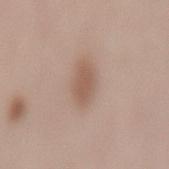{
  "biopsy_status": "not biopsied; imaged during a skin examination",
  "lighting": "white-light",
  "image": {
    "source": "total-body photography crop",
    "field_of_view_mm": 15
  },
  "site": "mid back",
  "lesion_size": {
    "long_diameter_mm_approx": 3.0
  },
  "patient": {
    "sex": "female",
    "age_approx": 40
  }
}